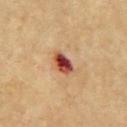Clinical impression: Captured during whole-body skin photography for melanoma surveillance; the lesion was not biopsied. Context: Located on the front of the torso. The tile uses cross-polarized illumination. The total-body-photography lesion software estimated an eccentricity of roughly 0.8 and a shape-asymmetry score of about 0.2 (0 = symmetric). The software also gave internal color variation of about 9.5 on a 0–10 scale. The analysis additionally found a classifier nevus-likeness of about 0/100 and a lesion-detection confidence of about 100/100. A 15 mm crop from a total-body photograph taken for skin-cancer surveillance. A male subject, roughly 65 years of age. The lesion's longest dimension is about 3.5 mm.